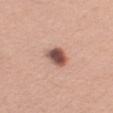Case summary:
• biopsy status — total-body-photography surveillance lesion; no biopsy
• acquisition — ~15 mm tile from a whole-body skin photo
• automated lesion analysis — an average lesion color of about L≈53 a*≈21 b*≈26 (CIELAB), a lesion–skin lightness drop of about 18, and a lesion-to-skin contrast of about 11.5 (normalized; higher = more distinct); a border-irregularity rating of about 1.5/10, a within-lesion color-variation index near 6.5/10, and a peripheral color-asymmetry measure near 2; a nevus-likeness score of about 95/100
• location — the right upper arm
• illumination — white-light
• lesion size — about 3 mm
• patient — male, in their 40s